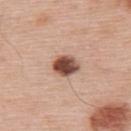| field | value |
|---|---|
| tile lighting | white-light illumination |
| imaging modality | ~15 mm tile from a whole-body skin photo |
| subject | male, roughly 60 years of age |
| automated metrics | a mean CIELAB color near L≈49 a*≈22 b*≈26, roughly 20 lightness units darker than nearby skin, and a lesion-to-skin contrast of about 13 (normalized; higher = more distinct); a color-variation rating of about 6/10 and radial color variation of about 2; a nevus-likeness score of about 100/100 and lesion-presence confidence of about 100/100 |
| size | about 3 mm |
| anatomic site | the upper back |